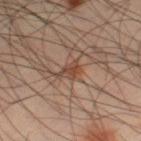notes: catalogued during a skin exam; not biopsied